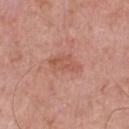Part of a total-body skin-imaging series; this lesion was reviewed on a skin check and was not flagged for biopsy.